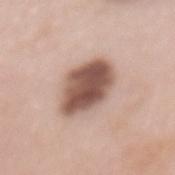{"biopsy_status": "not biopsied; imaged during a skin examination", "lesion_size": {"long_diameter_mm_approx": 5.5}, "image": {"source": "total-body photography crop", "field_of_view_mm": 15}, "automated_metrics": {"shape_asymmetry": 0.15, "cielab_L": 53, "cielab_a": 19, "cielab_b": 25, "vs_skin_contrast_norm": 12.0, "nevus_likeness_0_100": 65, "lesion_detection_confidence_0_100": 100}, "site": "back", "patient": {"sex": "female", "age_approx": 60}}